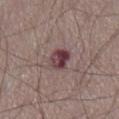Clinical impression:
The lesion was photographed on a routine skin check and not biopsied; there is no pathology result.
Context:
A male patient, aged around 75. Measured at roughly 3 mm in maximum diameter. The lesion is located on the abdomen. A roughly 15 mm field-of-view crop from a total-body skin photograph. Imaged with white-light lighting. The lesion-visualizer software estimated an average lesion color of about L≈40 a*≈21 b*≈14 (CIELAB), roughly 13 lightness units darker than nearby skin, and a normalized lesion–skin contrast near 11. The software also gave a color-variation rating of about 8.5/10 and radial color variation of about 3. The software also gave an automated nevus-likeness rating near 0 out of 100.